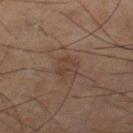Q: Was a biopsy performed?
A: catalogued during a skin exam; not biopsied
Q: What is the anatomic site?
A: the left thigh
Q: Automated lesion metrics?
A: a footprint of about 4 mm² and an outline eccentricity of about 0.6 (0 = round, 1 = elongated); an average lesion color of about L≈31 a*≈13 b*≈21 (CIELAB), roughly 4 lightness units darker than nearby skin, and a normalized border contrast of about 5
Q: Illumination type?
A: cross-polarized illumination
Q: What is the imaging modality?
A: total-body-photography crop, ~15 mm field of view
Q: What are the patient's age and sex?
A: male, in their mid- to late 60s